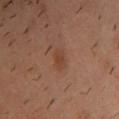Part of a total-body skin-imaging series; this lesion was reviewed on a skin check and was not flagged for biopsy.
The subject is a male aged 38 to 42.
Approximately 3 mm at its widest.
From the upper back.
A 15 mm crop from a total-body photograph taken for skin-cancer surveillance.
Imaged with cross-polarized lighting.